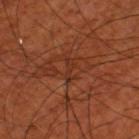This lesion was catalogued during total-body skin photography and was not selected for biopsy.